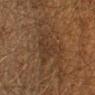The lesion was photographed on a routine skin check and not biopsied; there is no pathology result. Located on the left lower leg. Cropped from a whole-body photographic skin survey; the tile spans about 15 mm. A male patient, about 60 years old. The recorded lesion diameter is about 5.5 mm. This is a cross-polarized tile.